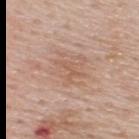Captured during whole-body skin photography for melanoma surveillance; the lesion was not biopsied. The lesion is on the upper back. Cropped from a whole-body photographic skin survey; the tile spans about 15 mm. The subject is a male aged 58–62.